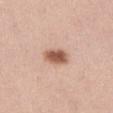Recorded during total-body skin imaging; not selected for excision or biopsy.
This image is a 15 mm lesion crop taken from a total-body photograph.
Imaged with white-light lighting.
On the left thigh.
The lesion's longest dimension is about 3.5 mm.
Automated image analysis of the tile measured a footprint of about 6.5 mm² and an eccentricity of roughly 0.8. The analysis additionally found a border-irregularity rating of about 2/10 and a within-lesion color-variation index near 3.5/10.
A female subject about 35 years old.